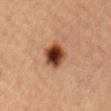<tbp_lesion>
<biopsy_status>not biopsied; imaged during a skin examination</biopsy_status>
<patient>
  <sex>female</sex>
  <age_approx>40</age_approx>
</patient>
<image>
  <source>total-body photography crop</source>
  <field_of_view_mm>15</field_of_view_mm>
</image>
<site>lower back</site>
</tbp_lesion>